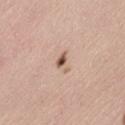Notes:
* notes · catalogued during a skin exam; not biopsied
* image · total-body-photography crop, ~15 mm field of view
* patient · female, aged 38 to 42
* anatomic site · the leg
* lesion size · ~3 mm (longest diameter)